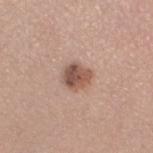Imaged during a routine full-body skin examination; the lesion was not biopsied and no histopathology is available.
Captured under white-light illumination.
The patient is a female aged approximately 45.
The lesion is on the leg.
A lesion tile, about 15 mm wide, cut from a 3D total-body photograph.